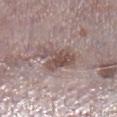Case summary:
* biopsy status: catalogued during a skin exam; not biopsied